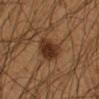Assessment:
Captured during whole-body skin photography for melanoma surveillance; the lesion was not biopsied.
Acquisition and patient details:
Longest diameter approximately 4 mm. This is a cross-polarized tile. Located on the left forearm. A region of skin cropped from a whole-body photographic capture, roughly 15 mm wide. A male patient, aged 38 to 42.